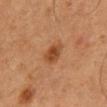notes — imaged on a skin check; not biopsied
TBP lesion metrics — a border-irregularity rating of about 2/10 and a within-lesion color-variation index near 3/10; a nevus-likeness score of about 85/100 and a lesion-detection confidence of about 100/100
image — ~15 mm crop, total-body skin-cancer survey
illumination — cross-polarized illumination
subject — male, aged 63 to 67
location — the mid back
lesion size — ~3 mm (longest diameter)